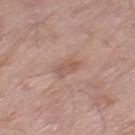Captured during whole-body skin photography for melanoma surveillance; the lesion was not biopsied. On the leg. A male subject, aged 78–82. This is a white-light tile. This image is a 15 mm lesion crop taken from a total-body photograph. An algorithmic analysis of the crop reported a footprint of about 5 mm², an eccentricity of roughly 0.7, and a symmetry-axis asymmetry near 0.25.